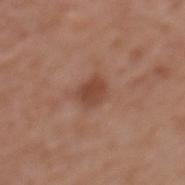Notes:
- biopsy status: catalogued during a skin exam; not biopsied
- illumination: white-light illumination
- acquisition: ~15 mm tile from a whole-body skin photo
- body site: the upper back
- lesion size: about 2.5 mm
- subject: female, aged 78–82